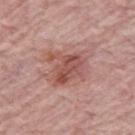Recorded during total-body skin imaging; not selected for excision or biopsy. Cropped from a total-body skin-imaging series; the visible field is about 15 mm. Longest diameter approximately 4.5 mm. Located on the right thigh. The tile uses white-light illumination. An algorithmic analysis of the crop reported an average lesion color of about L≈51 a*≈25 b*≈25 (CIELAB), a lesion–skin lightness drop of about 10, and a normalized lesion–skin contrast near 7. The patient is a female aged around 70.